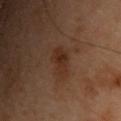biopsy_status: not biopsied; imaged during a skin examination
site: front of the torso
lesion_size:
  long_diameter_mm_approx: 4.0
lighting: cross-polarized
patient:
  sex: male
  age_approx: 55
image:
  source: total-body photography crop
  field_of_view_mm: 15